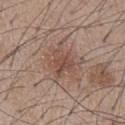Part of a total-body skin-imaging series; this lesion was reviewed on a skin check and was not flagged for biopsy.